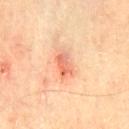Clinical impression: This lesion was catalogued during total-body skin photography and was not selected for biopsy. Image and clinical context: A male subject, aged approximately 65. About 3 mm across. Captured under cross-polarized illumination. The total-body-photography lesion software estimated an average lesion color of about L≈67 a*≈30 b*≈37 (CIELAB), about 11 CIELAB-L* units darker than the surrounding skin, and a normalized lesion–skin contrast near 6.5. And it measured an automated nevus-likeness rating near 5 out of 100. A 15 mm crop from a total-body photograph taken for skin-cancer surveillance. From the chest.